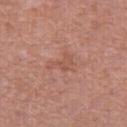image: total-body-photography crop, ~15 mm field of view | anatomic site: the right thigh | automated lesion analysis: border irregularity of about 6 on a 0–10 scale, a within-lesion color-variation index near 1.5/10, and radial color variation of about 0.5; a classifier nevus-likeness of about 0/100 and a lesion-detection confidence of about 100/100 | lighting: white-light | patient: female, approximately 40 years of age | lesion diameter: about 3.5 mm.